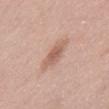notes: no biopsy performed (imaged during a skin exam) | image: 15 mm crop, total-body photography | patient: male, aged approximately 30 | lighting: white-light | site: the abdomen | lesion diameter: about 4 mm.